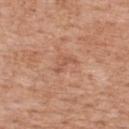The lesion was tiled from a total-body skin photograph and was not biopsied. A male subject, about 60 years old. A region of skin cropped from a whole-body photographic capture, roughly 15 mm wide. Approximately 2.5 mm at its widest. The lesion is located on the upper back.